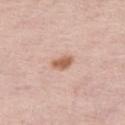* notes · imaged on a skin check; not biopsied